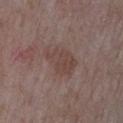| field | value |
|---|---|
| workup | total-body-photography surveillance lesion; no biopsy |
| illumination | white-light illumination |
| site | the left upper arm |
| image | ~15 mm tile from a whole-body skin photo |
| subject | male, about 65 years old |
| automated metrics | a lesion area of about 10 mm², an outline eccentricity of about 0.7 (0 = round, 1 = elongated), and a shape-asymmetry score of about 0.35 (0 = symmetric); an average lesion color of about L≈43 a*≈17 b*≈21 (CIELAB), a lesion–skin lightness drop of about 6, and a normalized lesion–skin contrast near 5.5 |